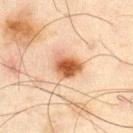notes = catalogued during a skin exam; not biopsied
subject = male, approximately 45 years of age
automated metrics = a mean CIELAB color near L≈49 a*≈21 b*≈33, about 14 CIELAB-L* units darker than the surrounding skin, and a normalized border contrast of about 10.5
image source = ~15 mm tile from a whole-body skin photo
site = the right thigh
lesion size = about 3.5 mm
illumination = cross-polarized illumination